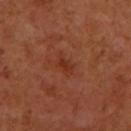Part of a total-body skin-imaging series; this lesion was reviewed on a skin check and was not flagged for biopsy.
On the arm.
Imaged with cross-polarized lighting.
Measured at roughly 2.5 mm in maximum diameter.
A roughly 15 mm field-of-view crop from a total-body skin photograph.
Automated image analysis of the tile measured a footprint of about 3.5 mm² and two-axis asymmetry of about 0.25. And it measured a lesion color around L≈35 a*≈29 b*≈32 in CIELAB and a normalized lesion–skin contrast near 6. And it measured internal color variation of about 2 on a 0–10 scale and peripheral color asymmetry of about 0.5. The software also gave a detector confidence of about 100 out of 100 that the crop contains a lesion.
A female subject in their 50s.